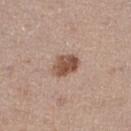Captured during whole-body skin photography for melanoma surveillance; the lesion was not biopsied.
Located on the leg.
Imaged with white-light lighting.
A region of skin cropped from a whole-body photographic capture, roughly 15 mm wide.
About 3.5 mm across.
The patient is a female aged 48–52.